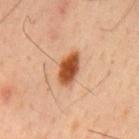The lesion was tiled from a total-body skin photograph and was not biopsied.
This is a cross-polarized tile.
A male subject roughly 45 years of age.
About 4 mm across.
From the mid back.
A roughly 15 mm field-of-view crop from a total-body skin photograph.